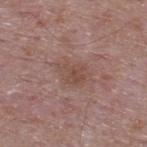{
  "biopsy_status": "not biopsied; imaged during a skin examination",
  "lighting": "white-light",
  "lesion_size": {
    "long_diameter_mm_approx": 4.0
  },
  "site": "upper back",
  "image": {
    "source": "total-body photography crop",
    "field_of_view_mm": 15
  },
  "patient": {
    "sex": "male",
    "age_approx": 65
  }
}